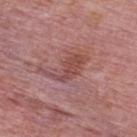| key | value |
|---|---|
| follow-up | no biopsy performed (imaged during a skin exam) |
| site | the upper back |
| patient | male, aged 73–77 |
| imaging modality | ~15 mm crop, total-body skin-cancer survey |